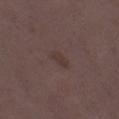No biopsy was performed on this lesion — it was imaged during a full skin examination and was not determined to be concerning.
A lesion tile, about 15 mm wide, cut from a 3D total-body photograph.
A female patient, in their mid- to late 30s.
The lesion is located on the left thigh.